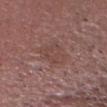The tile uses white-light illumination.
The total-body-photography lesion software estimated a footprint of about 9.5 mm², an eccentricity of roughly 0.8, and two-axis asymmetry of about 0.25. The analysis additionally found a lesion color around L≈45 a*≈20 b*≈22 in CIELAB, about 5 CIELAB-L* units darker than the surrounding skin, and a normalized border contrast of about 4.5.
The lesion's longest dimension is about 4.5 mm.
A 15 mm crop from a total-body photograph taken for skin-cancer surveillance.
From the head or neck.
The subject is a male aged approximately 55.